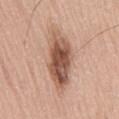| key | value |
|---|---|
| diameter | ≈7.5 mm |
| patient | male, aged 73 to 77 |
| illumination | white-light |
| location | the lower back |
| image source | ~15 mm tile from a whole-body skin photo |
| automated lesion analysis | two-axis asymmetry of about 0.2; border irregularity of about 3 on a 0–10 scale and a within-lesion color-variation index near 8/10; a lesion-detection confidence of about 100/100 |